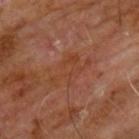Background: A male subject, approximately 60 years of age. Imaged with cross-polarized lighting. The recorded lesion diameter is about 5 mm. On the chest. This image is a 15 mm lesion crop taken from a total-body photograph.